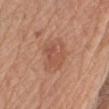The recorded lesion diameter is about 4.5 mm. On the right upper arm. The patient is a male in their 70s. This is a white-light tile. The total-body-photography lesion software estimated a shape eccentricity near 0.8 and a symmetry-axis asymmetry near 0.25. It also reported a mean CIELAB color near L≈53 a*≈24 b*≈31, roughly 8 lightness units darker than nearby skin, and a lesion-to-skin contrast of about 5.5 (normalized; higher = more distinct). The software also gave border irregularity of about 3 on a 0–10 scale and peripheral color asymmetry of about 1. A close-up tile cropped from a whole-body skin photograph, about 15 mm across.